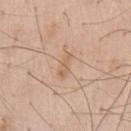Image and clinical context:
A male subject aged 58 to 62. Located on the chest. Measured at roughly 3 mm in maximum diameter. Automated tile analysis of the lesion measured a footprint of about 4 mm² and a shape eccentricity near 0.9. The software also gave a border-irregularity rating of about 2.5/10, internal color variation of about 1.5 on a 0–10 scale, and peripheral color asymmetry of about 0.5. It also reported a nevus-likeness score of about 0/100. A lesion tile, about 15 mm wide, cut from a 3D total-body photograph. Captured under white-light illumination.